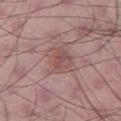Imaged during a routine full-body skin examination; the lesion was not biopsied and no histopathology is available. The lesion-visualizer software estimated a footprint of about 9.5 mm² and an outline eccentricity of about 0.6 (0 = round, 1 = elongated). It also reported an automated nevus-likeness rating near 10 out of 100 and a lesion-detection confidence of about 100/100. The tile uses white-light illumination. A male subject aged approximately 50. A close-up tile cropped from a whole-body skin photograph, about 15 mm across. The lesion's longest dimension is about 3.5 mm. The lesion is located on the right thigh.